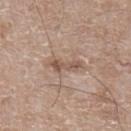follow-up: imaged on a skin check; not biopsied | body site: the left lower leg | acquisition: total-body-photography crop, ~15 mm field of view | subject: male, aged 63–67.